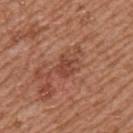Q: What kind of image is this?
A: ~15 mm crop, total-body skin-cancer survey
Q: How was the tile lit?
A: white-light illumination
Q: Who is the patient?
A: male, about 55 years old
Q: Where on the body is the lesion?
A: the upper back
Q: What did automated image analysis measure?
A: a lesion area of about 5 mm² and two-axis asymmetry of about 0.25; a lesion color around L≈45 a*≈25 b*≈30 in CIELAB, a lesion–skin lightness drop of about 8, and a normalized lesion–skin contrast near 6.5; a nevus-likeness score of about 0/100